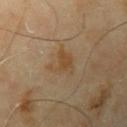Assessment:
The lesion was photographed on a routine skin check and not biopsied; there is no pathology result.
Acquisition and patient details:
Captured under cross-polarized illumination. The lesion is on the left upper arm. A lesion tile, about 15 mm wide, cut from a 3D total-body photograph. A male subject, in their mid-60s. Measured at roughly 3.5 mm in maximum diameter. The total-body-photography lesion software estimated a footprint of about 6 mm², an outline eccentricity of about 0.55 (0 = round, 1 = elongated), and two-axis asymmetry of about 0.4. The analysis additionally found peripheral color asymmetry of about 0.5.